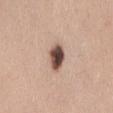Acquisition and patient details: Imaged with white-light lighting. A female subject roughly 40 years of age. Located on the lower back. A 15 mm close-up extracted from a 3D total-body photography capture. The recorded lesion diameter is about 3.5 mm. The total-body-photography lesion software estimated an automated nevus-likeness rating near 95 out of 100 and lesion-presence confidence of about 100/100.